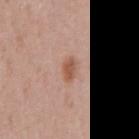Clinical impression: The lesion was photographed on a routine skin check and not biopsied; there is no pathology result. Clinical summary: A male patient, aged 53 to 57. Captured under white-light illumination. This image is a 15 mm lesion crop taken from a total-body photograph. The lesion is on the chest.